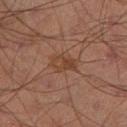Notes:
- follow-up — catalogued during a skin exam; not biopsied
- lighting — cross-polarized
- patient — male, aged around 70
- image source — ~15 mm crop, total-body skin-cancer survey
- lesion diameter — about 3.5 mm
- anatomic site — the left thigh